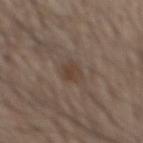* follow-up: imaged on a skin check; not biopsied
* image: total-body-photography crop, ~15 mm field of view
* location: the mid back
* subject: male, aged 58–62
* lesion diameter: ≈2.5 mm
* lighting: white-light illumination